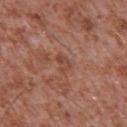• notes · total-body-photography surveillance lesion; no biopsy
• patient · male, about 45 years old
• image source · ~15 mm crop, total-body skin-cancer survey
• location · the front of the torso
• diameter · ~2.5 mm (longest diameter)